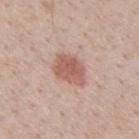<tbp_lesion>
<biopsy_status>not biopsied; imaged during a skin examination</biopsy_status>
<patient>
  <sex>male</sex>
  <age_approx>40</age_approx>
</patient>
<site>mid back</site>
<image>
  <source>total-body photography crop</source>
  <field_of_view_mm>15</field_of_view_mm>
</image>
</tbp_lesion>